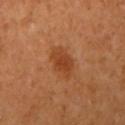Q: Was a biopsy performed?
A: total-body-photography surveillance lesion; no biopsy